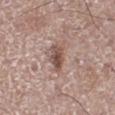| key | value |
|---|---|
| workup | total-body-photography surveillance lesion; no biopsy |
| location | the left lower leg |
| tile lighting | white-light illumination |
| size | about 3.5 mm |
| imaging modality | ~15 mm tile from a whole-body skin photo |
| subject | male, aged approximately 65 |